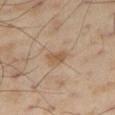Assessment:
Captured during whole-body skin photography for melanoma surveillance; the lesion was not biopsied.
Image and clinical context:
A roughly 15 mm field-of-view crop from a total-body skin photograph. The lesion's longest dimension is about 2.5 mm. A male patient roughly 55 years of age. Located on the right thigh.